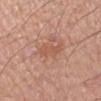Assessment:
The lesion was tiled from a total-body skin photograph and was not biopsied.
Background:
A region of skin cropped from a whole-body photographic capture, roughly 15 mm wide. A male patient in their 60s. The lesion-visualizer software estimated a footprint of about 4.5 mm² and an eccentricity of roughly 0.95. The analysis additionally found a normalized border contrast of about 5.5. The software also gave a border-irregularity rating of about 4/10, a color-variation rating of about 1/10, and a peripheral color-asymmetry measure near 0.5. Imaged with white-light lighting. Measured at roughly 3.5 mm in maximum diameter. The lesion is located on the left forearm.